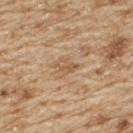Impression: The lesion was photographed on a routine skin check and not biopsied; there is no pathology result. Acquisition and patient details: The recorded lesion diameter is about 2.5 mm. Cropped from a whole-body photographic skin survey; the tile spans about 15 mm. A male subject roughly 70 years of age. Imaged with white-light lighting. The lesion is located on the upper back.